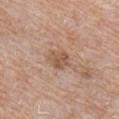follow-up — total-body-photography surveillance lesion; no biopsy | size — ≈2.5 mm | lighting — white-light illumination | image-analysis metrics — an area of roughly 5 mm² and a shape eccentricity near 0.55; a border-irregularity rating of about 2.5/10, a within-lesion color-variation index near 2.5/10, and a peripheral color-asymmetry measure near 1; a classifier nevus-likeness of about 20/100 and lesion-presence confidence of about 100/100 | subject — male, aged approximately 60 | anatomic site — the right upper arm | acquisition — ~15 mm crop, total-body skin-cancer survey.